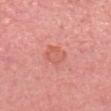  biopsy_status: not biopsied; imaged during a skin examination
  lesion_size:
    long_diameter_mm_approx: 3.0
  patient:
    sex: female
    age_approx: 40
  image:
    source: total-body photography crop
    field_of_view_mm: 15
  site: head or neck